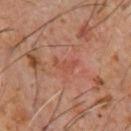Notes:
* patient: male, roughly 60 years of age
* illumination: cross-polarized
* diameter: about 2.5 mm
* body site: the front of the torso
* acquisition: ~15 mm tile from a whole-body skin photo